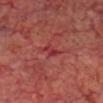The lesion was tiled from a total-body skin photograph and was not biopsied. About 2.5 mm across. The lesion-visualizer software estimated a lesion color around L≈36 a*≈35 b*≈23 in CIELAB, a lesion–skin lightness drop of about 7, and a normalized border contrast of about 6.5. And it measured internal color variation of about 0 on a 0–10 scale. And it measured a classifier nevus-likeness of about 0/100 and a detector confidence of about 75 out of 100 that the crop contains a lesion. From the head or neck. A close-up tile cropped from a whole-body skin photograph, about 15 mm across. A male patient, aged 63 to 67. The tile uses cross-polarized illumination.